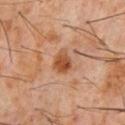The lesion was tiled from a total-body skin photograph and was not biopsied. A male subject in their 60s. Cropped from a total-body skin-imaging series; the visible field is about 15 mm. Located on the front of the torso. An algorithmic analysis of the crop reported a lesion area of about 5 mm². The software also gave a border-irregularity index near 1.5/10, a color-variation rating of about 3.5/10, and radial color variation of about 1. The analysis additionally found a nevus-likeness score of about 90/100 and a lesion-detection confidence of about 100/100. Approximately 3 mm at its widest.